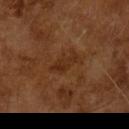Part of a total-body skin-imaging series; this lesion was reviewed on a skin check and was not flagged for biopsy.
A male subject in their mid-60s.
A 15 mm close-up extracted from a 3D total-body photography capture.
Approximately 3.5 mm at its widest.
This is a cross-polarized tile.
The total-body-photography lesion software estimated a mean CIELAB color near L≈28 a*≈21 b*≈31, about 6 CIELAB-L* units darker than the surrounding skin, and a normalized border contrast of about 6. It also reported border irregularity of about 4.5 on a 0–10 scale, a within-lesion color-variation index near 1/10, and radial color variation of about 0.5. It also reported a nevus-likeness score of about 0/100.